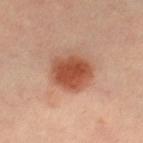Q: Was this lesion biopsied?
A: no biopsy performed (imaged during a skin exam)
Q: What is the anatomic site?
A: the right thigh
Q: What kind of image is this?
A: 15 mm crop, total-body photography
Q: Who is the patient?
A: female, approximately 35 years of age